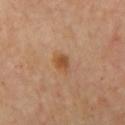A 15 mm close-up extracted from a 3D total-body photography capture. This is a cross-polarized tile. The recorded lesion diameter is about 2 mm. A female patient, in their mid- to late 60s. The lesion is on the right upper arm.